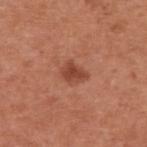workup = imaged on a skin check; not biopsied
imaging modality = 15 mm crop, total-body photography
lesion diameter = ~3 mm (longest diameter)
patient = male, about 55 years old
tile lighting = white-light
site = the upper back